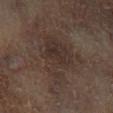This lesion was catalogued during total-body skin photography and was not selected for biopsy. A roughly 15 mm field-of-view crop from a total-body skin photograph. About 7.5 mm across. Captured under cross-polarized illumination. A male patient, aged 63–67. The lesion is located on the left lower leg.